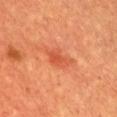Clinical impression: Captured during whole-body skin photography for melanoma surveillance; the lesion was not biopsied.